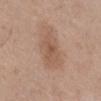The tile uses white-light illumination. A female patient, in their mid- to late 50s. Approximately 4.5 mm at its widest. Automated tile analysis of the lesion measured a lesion area of about 11 mm², an eccentricity of roughly 0.8, and two-axis asymmetry of about 0.25. It also reported an average lesion color of about L≈55 a*≈18 b*≈29 (CIELAB), roughly 8 lightness units darker than nearby skin, and a lesion-to-skin contrast of about 5.5 (normalized; higher = more distinct). From the right lower leg. A 15 mm close-up extracted from a 3D total-body photography capture.